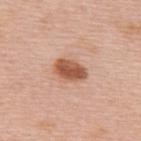The lesion was tiled from a total-body skin photograph and was not biopsied. The patient is a female aged 48 to 52. The lesion is on the upper back. A lesion tile, about 15 mm wide, cut from a 3D total-body photograph. This is a white-light tile. Longest diameter approximately 4 mm.